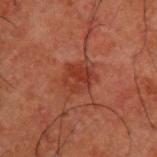This lesion was catalogued during total-body skin photography and was not selected for biopsy. The lesion is on the upper back. A region of skin cropped from a whole-body photographic capture, roughly 15 mm wide. A male patient, aged 48–52. Imaged with cross-polarized lighting. Automated tile analysis of the lesion measured peripheral color asymmetry of about 1.